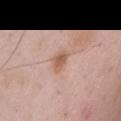The lesion was photographed on a routine skin check and not biopsied; there is no pathology result.
The tile uses white-light illumination.
Approximately 3 mm at its widest.
A male patient in their mid- to late 50s.
An algorithmic analysis of the crop reported an average lesion color of about L≈58 a*≈21 b*≈29 (CIELAB), roughly 10 lightness units darker than nearby skin, and a normalized border contrast of about 7.5.
A close-up tile cropped from a whole-body skin photograph, about 15 mm across.
Located on the chest.